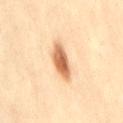Part of a total-body skin-imaging series; this lesion was reviewed on a skin check and was not flagged for biopsy.
From the lower back.
Measured at roughly 4.5 mm in maximum diameter.
The lesion-visualizer software estimated an average lesion color of about L≈58 a*≈20 b*≈35 (CIELAB) and a normalized border contrast of about 10.
A female patient, about 45 years old.
Captured under cross-polarized illumination.
A 15 mm crop from a total-body photograph taken for skin-cancer surveillance.